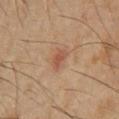• biopsy status — imaged on a skin check; not biopsied